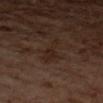Located on the left forearm. Longest diameter approximately 4 mm. This is a cross-polarized tile. The patient is a male aged approximately 60. Automated tile analysis of the lesion measured a lesion area of about 4.5 mm², an outline eccentricity of about 0.95 (0 = round, 1 = elongated), and two-axis asymmetry of about 0.5. The analysis additionally found an average lesion color of about L≈20 a*≈14 b*≈21 (CIELAB), roughly 4 lightness units darker than nearby skin, and a normalized border contrast of about 6. And it measured border irregularity of about 6 on a 0–10 scale, a color-variation rating of about 2/10, and peripheral color asymmetry of about 0.5. A region of skin cropped from a whole-body photographic capture, roughly 15 mm wide.